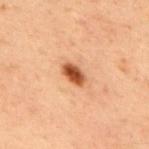Impression:
Part of a total-body skin-imaging series; this lesion was reviewed on a skin check and was not flagged for biopsy.
Background:
A male subject aged 58 to 62. On the upper back. A 15 mm crop from a total-body photograph taken for skin-cancer surveillance.